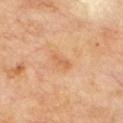Context: A region of skin cropped from a whole-body photographic capture, roughly 15 mm wide. This is a cross-polarized tile. On the chest. An algorithmic analysis of the crop reported a nevus-likeness score of about 0/100 and a lesion-detection confidence of about 100/100. The lesion's longest dimension is about 2.5 mm. The patient is a male aged around 70.